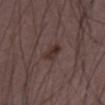Q: Was this lesion biopsied?
A: catalogued during a skin exam; not biopsied
Q: Illumination type?
A: white-light illumination
Q: What are the patient's age and sex?
A: male, approximately 50 years of age
Q: What is the anatomic site?
A: the left thigh
Q: Automated lesion metrics?
A: a classifier nevus-likeness of about 65/100 and a lesion-detection confidence of about 100/100
Q: How was this image acquired?
A: 15 mm crop, total-body photography